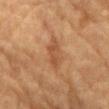Measured at roughly 4 mm in maximum diameter.
Automated tile analysis of the lesion measured a lesion area of about 6 mm², a shape eccentricity near 0.9, and a shape-asymmetry score of about 0.2 (0 = symmetric). The software also gave an average lesion color of about L≈44 a*≈20 b*≈31 (CIELAB), a lesion–skin lightness drop of about 7, and a normalized border contrast of about 5.5. It also reported lesion-presence confidence of about 100/100.
On the chest.
The subject is a male aged around 85 or older.
This is a cross-polarized tile.
A 15 mm close-up tile from a total-body photography series done for melanoma screening.